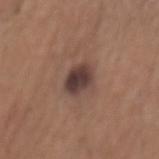No biopsy was performed on this lesion — it was imaged during a full skin examination and was not determined to be concerning.
Automated image analysis of the tile measured a mean CIELAB color near L≈39 a*≈17 b*≈20, about 12 CIELAB-L* units darker than the surrounding skin, and a normalized lesion–skin contrast near 11. And it measured a classifier nevus-likeness of about 10/100 and a detector confidence of about 100 out of 100 that the crop contains a lesion.
A 15 mm crop from a total-body photograph taken for skin-cancer surveillance.
On the back.
A male subject, about 65 years old.
Imaged with white-light lighting.
About 3.5 mm across.